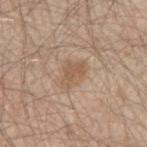Q: Was a biopsy performed?
A: no biopsy performed (imaged during a skin exam)
Q: What is the imaging modality?
A: ~15 mm tile from a whole-body skin photo
Q: What is the anatomic site?
A: the right forearm
Q: Who is the patient?
A: male, about 35 years old